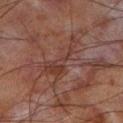workup: no biopsy performed (imaged during a skin exam); site: the left lower leg; patient: male, in their 70s; image source: 15 mm crop, total-body photography.